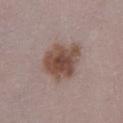Notes:
– notes: total-body-photography surveillance lesion; no biopsy
– image: ~15 mm tile from a whole-body skin photo
– tile lighting: white-light
– site: the left lower leg
– patient: male, in their 80s
– lesion size: ≈5 mm